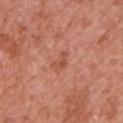<tbp_lesion>
  <biopsy_status>not biopsied; imaged during a skin examination</biopsy_status>
  <lighting>white-light</lighting>
  <patient>
    <sex>male</sex>
    <age_approx>65</age_approx>
  </patient>
  <image>
    <source>total-body photography crop</source>
    <field_of_view_mm>15</field_of_view_mm>
  </image>
  <site>left upper arm</site>
</tbp_lesion>